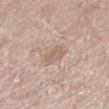Part of a total-body skin-imaging series; this lesion was reviewed on a skin check and was not flagged for biopsy.
A female subject, roughly 65 years of age.
On the right lower leg.
Cropped from a whole-body photographic skin survey; the tile spans about 15 mm.